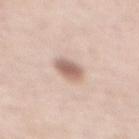biopsy_status: not biopsied; imaged during a skin examination
patient:
  sex: male
  age_approx: 60
lesion_size:
  long_diameter_mm_approx: 3.0
site: chest
automated_metrics:
  area_mm2_approx: 6.0
  eccentricity: 0.65
  shape_asymmetry: 0.1
  border_irregularity_0_10: 1.0
  color_variation_0_10: 3.5
  peripheral_color_asymmetry: 1.0
  nevus_likeness_0_100: 90
  lesion_detection_confidence_0_100: 100
image:
  source: total-body photography crop
  field_of_view_mm: 15